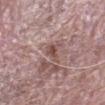Assessment:
Recorded during total-body skin imaging; not selected for excision or biopsy.
Clinical summary:
The tile uses white-light illumination. A male patient aged approximately 65. The total-body-photography lesion software estimated a lesion area of about 3.5 mm², an outline eccentricity of about 0.9 (0 = round, 1 = elongated), and a shape-asymmetry score of about 0.45 (0 = symmetric). The software also gave a lesion color around L≈49 a*≈20 b*≈21 in CIELAB, a lesion–skin lightness drop of about 10, and a normalized lesion–skin contrast near 7.5. It also reported a nevus-likeness score of about 0/100. The lesion is located on the left forearm. A 15 mm close-up extracted from a 3D total-body photography capture. About 3 mm across.